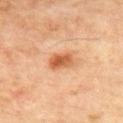Impression:
Captured during whole-body skin photography for melanoma surveillance; the lesion was not biopsied.
Clinical summary:
On the mid back. Captured under cross-polarized illumination. Automated tile analysis of the lesion measured a footprint of about 6 mm², an eccentricity of roughly 0.75, and a shape-asymmetry score of about 0.2 (0 = symmetric). The software also gave a mean CIELAB color near L≈56 a*≈25 b*≈39 and a normalized border contrast of about 8.5. And it measured a border-irregularity index near 2/10, a color-variation rating of about 5.5/10, and radial color variation of about 2. The software also gave a lesion-detection confidence of about 100/100. A roughly 15 mm field-of-view crop from a total-body skin photograph. The patient is a male aged 53 to 57. Longest diameter approximately 3 mm.